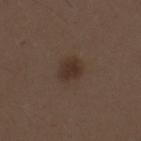Impression:
This lesion was catalogued during total-body skin photography and was not selected for biopsy.
Clinical summary:
Captured under white-light illumination. A roughly 15 mm field-of-view crop from a total-body skin photograph. The total-body-photography lesion software estimated a footprint of about 5.5 mm², an eccentricity of roughly 0.3, and a shape-asymmetry score of about 0.25 (0 = symmetric). The analysis additionally found an average lesion color of about L≈31 a*≈14 b*≈22 (CIELAB), roughly 7 lightness units darker than nearby skin, and a normalized border contrast of about 7.5. The analysis additionally found border irregularity of about 2 on a 0–10 scale, a within-lesion color-variation index near 2/10, and radial color variation of about 0.5. A male patient, aged around 30. The lesion is on the right upper arm. Longest diameter approximately 2.5 mm.